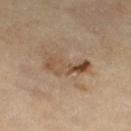biopsy status=catalogued during a skin exam; not biopsied
tile lighting=cross-polarized illumination
patient=male, aged around 65
image=total-body-photography crop, ~15 mm field of view
site=the right thigh